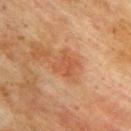{"patient": {"sex": "male", "age_approx": 75}, "image": {"source": "total-body photography crop", "field_of_view_mm": 15}, "site": "upper back"}